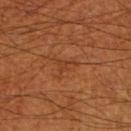tile lighting = cross-polarized
subject = male, aged around 70
image source = 15 mm crop, total-body photography
automated metrics = a lesion area of about 3.5 mm²
anatomic site = the right thigh